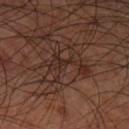Findings:
* illumination — cross-polarized
* patient — male, aged around 70
* acquisition — ~15 mm tile from a whole-body skin photo
* size — about 5.5 mm
* location — the left lower leg